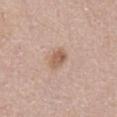{"biopsy_status": "not biopsied; imaged during a skin examination", "lesion_size": {"long_diameter_mm_approx": 2.5}, "image": {"source": "total-body photography crop", "field_of_view_mm": 15}, "lighting": "white-light", "site": "front of the torso", "patient": {"sex": "male", "age_approx": 75}}